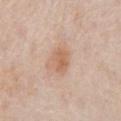Q: Was a biopsy performed?
A: imaged on a skin check; not biopsied
Q: How was the tile lit?
A: white-light illumination
Q: How large is the lesion?
A: about 3 mm
Q: Where on the body is the lesion?
A: the front of the torso
Q: What are the patient's age and sex?
A: male, aged approximately 75
Q: How was this image acquired?
A: ~15 mm tile from a whole-body skin photo
Q: Automated lesion metrics?
A: a shape eccentricity near 0.75 and a symmetry-axis asymmetry near 0.25; a lesion–skin lightness drop of about 9 and a normalized border contrast of about 6.5; a nevus-likeness score of about 70/100